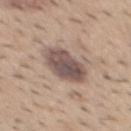biopsy status=catalogued during a skin exam; not biopsied | site=the mid back | TBP lesion metrics=internal color variation of about 4.5 on a 0–10 scale and peripheral color asymmetry of about 1.5 | image=~15 mm tile from a whole-body skin photo | patient=male, approximately 40 years of age | lighting=white-light.